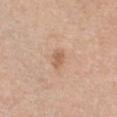Q: Is there a histopathology result?
A: catalogued during a skin exam; not biopsied
Q: Who is the patient?
A: female, about 40 years old
Q: Lesion size?
A: about 2.5 mm
Q: What lighting was used for the tile?
A: white-light
Q: Lesion location?
A: the front of the torso
Q: What is the imaging modality?
A: total-body-photography crop, ~15 mm field of view